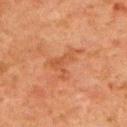Recorded during total-body skin imaging; not selected for excision or biopsy. Automated tile analysis of the lesion measured a footprint of about 8 mm² and an eccentricity of roughly 0.75. A lesion tile, about 15 mm wide, cut from a 3D total-body photograph. The lesion is located on the upper back. Measured at roughly 4.5 mm in maximum diameter. This is a cross-polarized tile. A male patient aged 78 to 82.